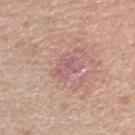Captured during whole-body skin photography for melanoma surveillance; the lesion was not biopsied.
Imaged with white-light lighting.
Approximately 3 mm at its widest.
On the right lower leg.
A female subject in their mid-60s.
Cropped from a whole-body photographic skin survey; the tile spans about 15 mm.